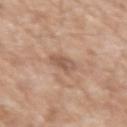biopsy status = total-body-photography surveillance lesion; no biopsy
anatomic site = the left forearm
image source = 15 mm crop, total-body photography
lesion size = ~2.5 mm (longest diameter)
patient = male, aged 68–72
illumination = white-light